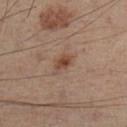Clinical summary: From the left leg. A male subject, about 50 years old. A close-up tile cropped from a whole-body skin photograph, about 15 mm across.